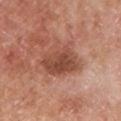Recorded during total-body skin imaging; not selected for excision or biopsy. A close-up tile cropped from a whole-body skin photograph, about 15 mm across. The lesion-visualizer software estimated a lesion area of about 16 mm², a shape eccentricity near 0.75, and two-axis asymmetry of about 0.2. The analysis additionally found a mean CIELAB color near L≈49 a*≈25 b*≈30 and a normalized lesion–skin contrast near 8. The software also gave a border-irregularity index near 3/10, a within-lesion color-variation index near 5/10, and radial color variation of about 2. On the left lower leg. This is a white-light tile. Measured at roughly 6 mm in maximum diameter. A male patient, aged 78–82.